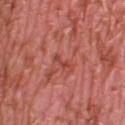{"biopsy_status": "not biopsied; imaged during a skin examination", "site": "leg", "lesion_size": {"long_diameter_mm_approx": 2.5}, "lighting": "white-light", "patient": {"sex": "female", "age_approx": 40}, "image": {"source": "total-body photography crop", "field_of_view_mm": 15}}